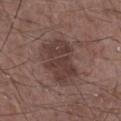The total-body-photography lesion software estimated a lesion area of about 15 mm² and an outline eccentricity of about 0.75 (0 = round, 1 = elongated). And it measured an automated nevus-likeness rating near 5 out of 100 and a detector confidence of about 100 out of 100 that the crop contains a lesion. A male patient, aged 58–62. A roughly 15 mm field-of-view crop from a total-body skin photograph. Measured at roughly 5.5 mm in maximum diameter. This is a white-light tile. Located on the chest.